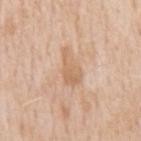Q: Was a biopsy performed?
A: total-body-photography surveillance lesion; no biopsy
Q: What lighting was used for the tile?
A: white-light
Q: What is the anatomic site?
A: the back
Q: What kind of image is this?
A: total-body-photography crop, ~15 mm field of view
Q: Who is the patient?
A: male, about 60 years old
Q: What is the lesion's diameter?
A: ≈4.5 mm